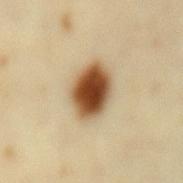Notes:
– workup — imaged on a skin check; not biopsied
– subject — female, roughly 35 years of age
– site — the mid back
– image — ~15 mm crop, total-body skin-cancer survey
– illumination — cross-polarized illumination